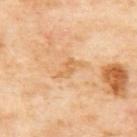Assessment: Captured during whole-body skin photography for melanoma surveillance; the lesion was not biopsied. Background: On the upper back. This is a cross-polarized tile. A female subject, in their 60s. Longest diameter approximately 3 mm. This image is a 15 mm lesion crop taken from a total-body photograph. An algorithmic analysis of the crop reported a footprint of about 3 mm², an outline eccentricity of about 0.85 (0 = round, 1 = elongated), and a shape-asymmetry score of about 0.4 (0 = symmetric). And it measured a mean CIELAB color near L≈66 a*≈21 b*≈43 and a lesion-to-skin contrast of about 5.5 (normalized; higher = more distinct). The software also gave a border-irregularity index near 4.5/10 and internal color variation of about 1 on a 0–10 scale. The analysis additionally found an automated nevus-likeness rating near 0 out of 100 and lesion-presence confidence of about 100/100.